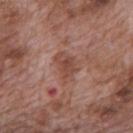Clinical impression: The lesion was photographed on a routine skin check and not biopsied; there is no pathology result. Background: About 4 mm across. The lesion-visualizer software estimated a mean CIELAB color near L≈47 a*≈22 b*≈27, roughly 8 lightness units darker than nearby skin, and a lesion-to-skin contrast of about 6.5 (normalized; higher = more distinct). It also reported a border-irregularity index near 3.5/10, a within-lesion color-variation index near 3/10, and radial color variation of about 1. It also reported an automated nevus-likeness rating near 0 out of 100 and lesion-presence confidence of about 100/100. A male patient, roughly 70 years of age. The lesion is on the mid back. This is a white-light tile. This image is a 15 mm lesion crop taken from a total-body photograph.